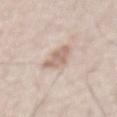biopsy_status: not biopsied; imaged during a skin examination
site: chest
image:
  source: total-body photography crop
  field_of_view_mm: 15
patient:
  sex: male
  age_approx: 50